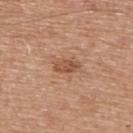Q: Was a biopsy performed?
A: total-body-photography surveillance lesion; no biopsy
Q: Lesion location?
A: the upper back
Q: What lighting was used for the tile?
A: white-light illumination
Q: Automated lesion metrics?
A: a lesion area of about 4 mm², an outline eccentricity of about 0.9 (0 = round, 1 = elongated), and a symmetry-axis asymmetry near 0.3; a lesion color around L≈52 a*≈23 b*≈33 in CIELAB, roughly 10 lightness units darker than nearby skin, and a lesion-to-skin contrast of about 7.5 (normalized; higher = more distinct); border irregularity of about 3 on a 0–10 scale, internal color variation of about 2.5 on a 0–10 scale, and radial color variation of about 1; an automated nevus-likeness rating near 50 out of 100 and a lesion-detection confidence of about 100/100
Q: What are the patient's age and sex?
A: male, aged 63–67
Q: What is the imaging modality?
A: 15 mm crop, total-body photography
Q: Lesion size?
A: ≈3 mm